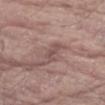Case summary:
- workup: total-body-photography surveillance lesion; no biopsy
- lighting: white-light
- diameter: ~3 mm (longest diameter)
- TBP lesion metrics: a footprint of about 4 mm² and two-axis asymmetry of about 0.35; border irregularity of about 4 on a 0–10 scale and a peripheral color-asymmetry measure near 0; a nevus-likeness score of about 0/100 and a lesion-detection confidence of about 80/100
- image source: ~15 mm crop, total-body skin-cancer survey
- body site: the right forearm
- patient: male, in their 80s